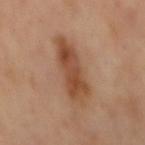Impression: This lesion was catalogued during total-body skin photography and was not selected for biopsy. Image and clinical context: The tile uses cross-polarized illumination. Approximately 8 mm at its widest. A 15 mm crop from a total-body photograph taken for skin-cancer surveillance. The total-body-photography lesion software estimated a border-irregularity rating of about 3/10, a within-lesion color-variation index near 5/10, and radial color variation of about 1.5. The software also gave an automated nevus-likeness rating near 40 out of 100 and lesion-presence confidence of about 100/100. From the mid back.